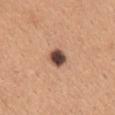TBP lesion metrics: an average lesion color of about L≈47 a*≈19 b*≈26 (CIELAB), about 20 CIELAB-L* units darker than the surrounding skin, and a normalized border contrast of about 14; border irregularity of about 1.5 on a 0–10 scale, internal color variation of about 6.5 on a 0–10 scale, and radial color variation of about 1.5; a classifier nevus-likeness of about 75/100 and a detector confidence of about 100 out of 100 that the crop contains a lesion
image: ~15 mm crop, total-body skin-cancer survey
body site: the mid back
lesion size: ~2.5 mm (longest diameter)
patient: female, aged 28 to 32